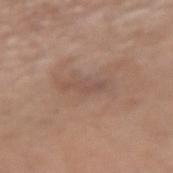Impression: Part of a total-body skin-imaging series; this lesion was reviewed on a skin check and was not flagged for biopsy. Background: Longest diameter approximately 4 mm. The patient is a male aged around 80. Cropped from a total-body skin-imaging series; the visible field is about 15 mm. The lesion is located on the arm.